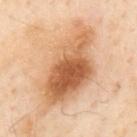This is a cross-polarized tile. A male subject, in their mid-50s. The lesion is on the mid back. A close-up tile cropped from a whole-body skin photograph, about 15 mm across.